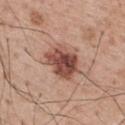Impression: The lesion was photographed on a routine skin check and not biopsied; there is no pathology result. Context: The lesion is located on the upper back. Approximately 4.5 mm at its widest. A roughly 15 mm field-of-view crop from a total-body skin photograph. A male subject about 65 years old.